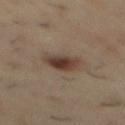No biopsy was performed on this lesion — it was imaged during a full skin examination and was not determined to be concerning. This image is a 15 mm lesion crop taken from a total-body photograph. The patient is a male in their mid- to late 50s. On the mid back.